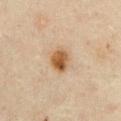workup: catalogued during a skin exam; not biopsied
acquisition: ~15 mm crop, total-body skin-cancer survey
anatomic site: the chest
lesion diameter: ~3.5 mm (longest diameter)
lighting: cross-polarized illumination
patient: male, about 45 years old